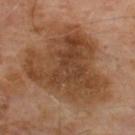This lesion was catalogued during total-body skin photography and was not selected for biopsy.
Longest diameter approximately 10 mm.
The lesion is on the upper back.
A male patient aged 63 to 67.
Captured under cross-polarized illumination.
Cropped from a whole-body photographic skin survey; the tile spans about 15 mm.